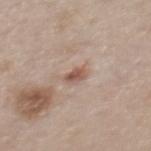| feature | finding |
|---|---|
| workup | total-body-photography surveillance lesion; no biopsy |
| body site | the mid back |
| lesion size | ≈2.5 mm |
| tile lighting | white-light |
| imaging modality | 15 mm crop, total-body photography |
| patient | female, roughly 40 years of age |